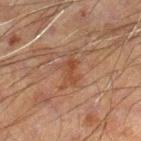automated_metrics:
  area_mm2_approx: 6.0
  shape_asymmetry: 0.5
  border_irregularity_0_10: 8.0
  nevus_likeness_0_100: 0
  lesion_detection_confidence_0_100: 100
site: left leg
patient:
  sex: male
  age_approx: 60
lesion_size:
  long_diameter_mm_approx: 4.0
image:
  source: total-body photography crop
  field_of_view_mm: 15
lighting: cross-polarized